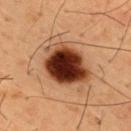Clinical impression:
The lesion was tiled from a total-body skin photograph and was not biopsied.
Background:
A male subject, about 55 years old. This image is a 15 mm lesion crop taken from a total-body photograph. Automated tile analysis of the lesion measured an area of roughly 21 mm² and a shape eccentricity near 0.65. The software also gave a lesion color around L≈31 a*≈23 b*≈28 in CIELAB, about 22 CIELAB-L* units darker than the surrounding skin, and a lesion-to-skin contrast of about 18 (normalized; higher = more distinct). It also reported border irregularity of about 2 on a 0–10 scale, a within-lesion color-variation index near 9.5/10, and a peripheral color-asymmetry measure near 3. It also reported an automated nevus-likeness rating near 95 out of 100 and lesion-presence confidence of about 100/100. Located on the chest. About 6.5 mm across. Captured under cross-polarized illumination.